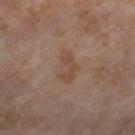• follow-up · no biopsy performed (imaged during a skin exam)
• image source · ~15 mm crop, total-body skin-cancer survey
• anatomic site · the right thigh
• diameter · about 4 mm
• patient · female, aged around 55